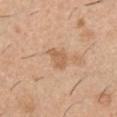Part of a total-body skin-imaging series; this lesion was reviewed on a skin check and was not flagged for biopsy. On the right upper arm. This is a white-light tile. The patient is a male in their 40s. The lesion-visualizer software estimated an area of roughly 4.5 mm² and a shape-asymmetry score of about 0.25 (0 = symmetric). It also reported a mean CIELAB color near L≈60 a*≈20 b*≈35, about 9 CIELAB-L* units darker than the surrounding skin, and a lesion-to-skin contrast of about 6 (normalized; higher = more distinct). And it measured a within-lesion color-variation index near 1.5/10 and radial color variation of about 0.5. And it measured a classifier nevus-likeness of about 0/100 and lesion-presence confidence of about 100/100. The lesion's longest dimension is about 3 mm. Cropped from a whole-body photographic skin survey; the tile spans about 15 mm.